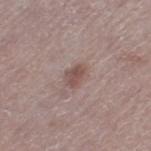workup: catalogued during a skin exam; not biopsied
lighting: white-light
subject: female, about 65 years old
anatomic site: the left thigh
automated metrics: an area of roughly 4 mm² and an eccentricity of roughly 0.75; an average lesion color of about L≈50 a*≈18 b*≈21 (CIELAB), a lesion–skin lightness drop of about 10, and a lesion-to-skin contrast of about 7 (normalized; higher = more distinct)
image: ~15 mm tile from a whole-body skin photo
size: ~2.5 mm (longest diameter)